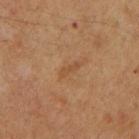Case summary:
• workup — total-body-photography surveillance lesion; no biopsy
• subject — male, roughly 60 years of age
• tile lighting — cross-polarized illumination
• anatomic site — the mid back
• imaging modality — ~15 mm crop, total-body skin-cancer survey
• diameter — ~2.5 mm (longest diameter)